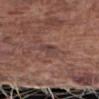The lesion was photographed on a routine skin check and not biopsied; there is no pathology result. The lesion-visualizer software estimated a lesion color around L≈41 a*≈18 b*≈20 in CIELAB and a normalized lesion–skin contrast near 6.5. The analysis additionally found a border-irregularity rating of about 3/10, a color-variation rating of about 5/10, and a peripheral color-asymmetry measure near 2. And it measured an automated nevus-likeness rating near 0 out of 100 and a lesion-detection confidence of about 90/100. A lesion tile, about 15 mm wide, cut from a 3D total-body photograph. The lesion's longest dimension is about 2.5 mm. From the right forearm. A male patient, in their mid- to late 70s.